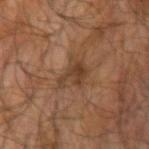The lesion was tiled from a total-body skin photograph and was not biopsied. A male subject, aged approximately 65. A roughly 15 mm field-of-view crop from a total-body skin photograph. Captured under cross-polarized illumination. The lesion is located on the right upper arm. Automated tile analysis of the lesion measured roughly 8 lightness units darker than nearby skin and a normalized border contrast of about 7.